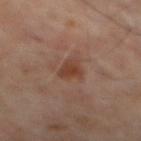No biopsy was performed on this lesion — it was imaged during a full skin examination and was not determined to be concerning.
This is a cross-polarized tile.
The subject is a male aged around 65.
Approximately 3 mm at its widest.
A 15 mm close-up extracted from a 3D total-body photography capture.
The total-body-photography lesion software estimated an automated nevus-likeness rating near 55 out of 100 and lesion-presence confidence of about 100/100.
From the mid back.